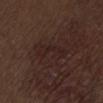The lesion was photographed on a routine skin check and not biopsied; there is no pathology result. The lesion is on the lower back. The recorded lesion diameter is about 4 mm. A male patient, approximately 70 years of age. Automated tile analysis of the lesion measured a lesion color around L≈21 a*≈17 b*≈17 in CIELAB, roughly 4 lightness units darker than nearby skin, and a normalized border contrast of about 5. This image is a 15 mm lesion crop taken from a total-body photograph. Imaged with white-light lighting.